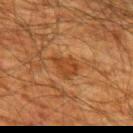The lesion was tiled from a total-body skin photograph and was not biopsied. Cropped from a whole-body photographic skin survey; the tile spans about 15 mm. Automated tile analysis of the lesion measured a mean CIELAB color near L≈33 a*≈21 b*≈33 and roughly 7 lightness units darker than nearby skin. The analysis additionally found a border-irregularity rating of about 2.5/10, a within-lesion color-variation index near 2.5/10, and peripheral color asymmetry of about 1. And it measured a classifier nevus-likeness of about 0/100 and a lesion-detection confidence of about 100/100. The lesion is on the back. The patient is a male about 60 years old.